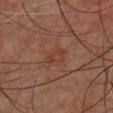Clinical impression: Recorded during total-body skin imaging; not selected for excision or biopsy. Context: The tile uses cross-polarized illumination. The recorded lesion diameter is about 3.5 mm. The patient is a male aged 43–47. On the chest. Cropped from a total-body skin-imaging series; the visible field is about 15 mm.